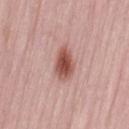Assessment: Part of a total-body skin-imaging series; this lesion was reviewed on a skin check and was not flagged for biopsy. Clinical summary: A close-up tile cropped from a whole-body skin photograph, about 15 mm across. A female subject, aged around 50. The lesion is on the lower back. The tile uses white-light illumination.